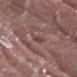Assessment:
Imaged during a routine full-body skin examination; the lesion was not biopsied and no histopathology is available.
Image and clinical context:
From the lower back. The subject is a male aged 38 to 42. Imaged with white-light lighting. A 15 mm close-up extracted from a 3D total-body photography capture. Approximately 3 mm at its widest.